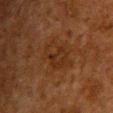Part of a total-body skin-imaging series; this lesion was reviewed on a skin check and was not flagged for biopsy. From the chest. The total-body-photography lesion software estimated a mean CIELAB color near L≈22 a*≈17 b*≈26 and a normalized lesion–skin contrast near 6. The software also gave border irregularity of about 3 on a 0–10 scale, a within-lesion color-variation index near 3.5/10, and a peripheral color-asymmetry measure near 1. This image is a 15 mm lesion crop taken from a total-body photograph. Imaged with cross-polarized lighting. A male subject, aged around 60. The recorded lesion diameter is about 5 mm.